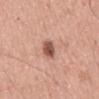biopsy status: total-body-photography surveillance lesion; no biopsy | automated lesion analysis: a nevus-likeness score of about 95/100 | patient: male, about 60 years old | illumination: white-light illumination | imaging modality: ~15 mm tile from a whole-body skin photo | lesion size: about 3.5 mm | location: the mid back.